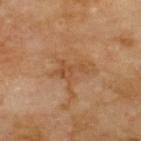<lesion>
  <site>back</site>
  <image>
    <source>total-body photography crop</source>
    <field_of_view_mm>15</field_of_view_mm>
  </image>
  <patient>
    <sex>male</sex>
    <age_approx>70</age_approx>
  </patient>
  <lesion_size>
    <long_diameter_mm_approx>3.5</long_diameter_mm_approx>
  </lesion_size>
</lesion>